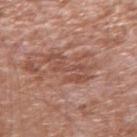notes = catalogued during a skin exam; not biopsied
patient = male, in their 60s
anatomic site = the right upper arm
illumination = white-light illumination
imaging modality = 15 mm crop, total-body photography
diameter = about 5.5 mm